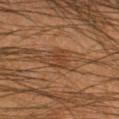Impression:
This lesion was catalogued during total-body skin photography and was not selected for biopsy.
Context:
Cropped from a whole-body photographic skin survey; the tile spans about 15 mm. On the left thigh. A male subject, about 35 years old. The recorded lesion diameter is about 3 mm. Imaged with cross-polarized lighting.